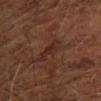workup: total-body-photography surveillance lesion; no biopsy
image: ~15 mm crop, total-body skin-cancer survey
TBP lesion metrics: a lesion color around L≈27 a*≈19 b*≈23 in CIELAB and a lesion-to-skin contrast of about 6 (normalized; higher = more distinct); a lesion-detection confidence of about 85/100
patient: male, approximately 65 years of age